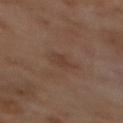A 15 mm close-up extracted from a 3D total-body photography capture.
An algorithmic analysis of the crop reported border irregularity of about 2.5 on a 0–10 scale, a within-lesion color-variation index near 1/10, and a peripheral color-asymmetry measure near 0.5. And it measured a classifier nevus-likeness of about 0/100 and a lesion-detection confidence of about 100/100.
This is a cross-polarized tile.
Approximately 2.5 mm at its widest.
The patient is a male aged 68–72.
The lesion is located on the back.